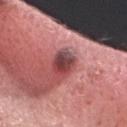The lesion was tiled from a total-body skin photograph and was not biopsied. Cropped from a total-body skin-imaging series; the visible field is about 15 mm. Located on the head or neck. A male patient, in their mid-30s.